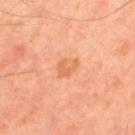This lesion was catalogued during total-body skin photography and was not selected for biopsy. On the upper back. A 15 mm crop from a total-body photograph taken for skin-cancer surveillance. Approximately 3 mm at its widest. Captured under cross-polarized illumination. A male subject aged 48–52. The lesion-visualizer software estimated an area of roughly 4.5 mm². It also reported a within-lesion color-variation index near 2/10 and radial color variation of about 1. It also reported a nevus-likeness score of about 10/100 and lesion-presence confidence of about 100/100.